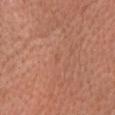Clinical impression: The lesion was photographed on a routine skin check and not biopsied; there is no pathology result. Acquisition and patient details: Located on the head or neck. The subject is a female aged approximately 55. A 15 mm close-up tile from a total-body photography series done for melanoma screening.